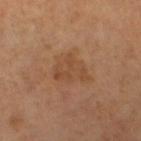Captured during whole-body skin photography for melanoma surveillance; the lesion was not biopsied. A close-up tile cropped from a whole-body skin photograph, about 15 mm across. Approximately 5 mm at its widest. Located on the left lower leg. The patient is a female in their mid- to late 50s.